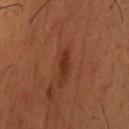No biopsy was performed on this lesion — it was imaged during a full skin examination and was not determined to be concerning.
The lesion is located on the head or neck.
The subject is a male aged 33 to 37.
Cropped from a whole-body photographic skin survey; the tile spans about 15 mm.